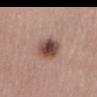* notes: no biopsy performed (imaged during a skin exam)
* site: the right thigh
* diameter: ≈4 mm
* lighting: white-light illumination
* image-analysis metrics: a shape eccentricity near 0.65 and two-axis asymmetry of about 0.15; a within-lesion color-variation index near 6/10 and peripheral color asymmetry of about 1.5; a nevus-likeness score of about 95/100 and lesion-presence confidence of about 100/100
* acquisition: total-body-photography crop, ~15 mm field of view
* patient: male, in their mid-70s